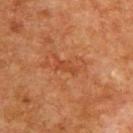Captured during whole-body skin photography for melanoma surveillance; the lesion was not biopsied.
The subject is a male aged around 65.
Cropped from a whole-body photographic skin survey; the tile spans about 15 mm.
From the upper back.
The tile uses cross-polarized illumination.